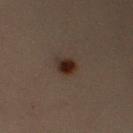Recorded during total-body skin imaging; not selected for excision or biopsy.
The lesion is located on the left arm.
This is a cross-polarized tile.
The subject is a female aged around 30.
The lesion-visualizer software estimated a lesion area of about 4.5 mm² and a shape-asymmetry score of about 0.15 (0 = symmetric). The analysis additionally found a lesion color around L≈20 a*≈12 b*≈17 in CIELAB, roughly 9 lightness units darker than nearby skin, and a normalized lesion–skin contrast near 11. The analysis additionally found border irregularity of about 1.5 on a 0–10 scale and a peripheral color-asymmetry measure near 2. The analysis additionally found an automated nevus-likeness rating near 100 out of 100 and a detector confidence of about 100 out of 100 that the crop contains a lesion.
This image is a 15 mm lesion crop taken from a total-body photograph.